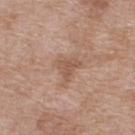biopsy status = no biopsy performed (imaged during a skin exam)
site = the upper back
illumination = white-light
patient = female, approximately 40 years of age
acquisition = ~15 mm crop, total-body skin-cancer survey